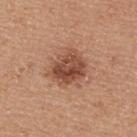The lesion was tiled from a total-body skin photograph and was not biopsied.
A region of skin cropped from a whole-body photographic capture, roughly 15 mm wide.
A male patient, roughly 40 years of age.
The total-body-photography lesion software estimated an eccentricity of roughly 0.45 and a symmetry-axis asymmetry near 0.2. It also reported a lesion color around L≈49 a*≈23 b*≈31 in CIELAB, roughly 12 lightness units darker than nearby skin, and a normalized border contrast of about 8.5. And it measured a classifier nevus-likeness of about 65/100.
The lesion is on the upper back.
Longest diameter approximately 4.5 mm.